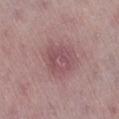{
  "biopsy_status": "not biopsied; imaged during a skin examination",
  "patient": {
    "sex": "female",
    "age_approx": 50
  },
  "site": "leg",
  "lighting": "white-light",
  "image": {
    "source": "total-body photography crop",
    "field_of_view_mm": 15
  }
}